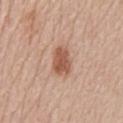<record>
<biopsy_status>not biopsied; imaged during a skin examination</biopsy_status>
<lighting>white-light</lighting>
<image>
  <source>total-body photography crop</source>
  <field_of_view_mm>15</field_of_view_mm>
</image>
<lesion_size>
  <long_diameter_mm_approx>4.0</long_diameter_mm_approx>
</lesion_size>
<patient>
  <sex>female</sex>
  <age_approx>45</age_approx>
</patient>
<site>front of the torso</site>
</record>